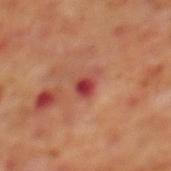Case summary:
- tile lighting: cross-polarized illumination
- size: ~3 mm (longest diameter)
- subject: male, in their 70s
- body site: the mid back
- acquisition: ~15 mm tile from a whole-body skin photo